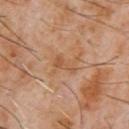No biopsy was performed on this lesion — it was imaged during a full skin examination and was not determined to be concerning. A 15 mm crop from a total-body photograph taken for skin-cancer surveillance. An algorithmic analysis of the crop reported border irregularity of about 6.5 on a 0–10 scale, a within-lesion color-variation index near 2/10, and peripheral color asymmetry of about 0.5. And it measured a classifier nevus-likeness of about 0/100 and lesion-presence confidence of about 100/100. Imaged with cross-polarized lighting. A male subject, aged around 60. About 3 mm across. The lesion is located on the chest.